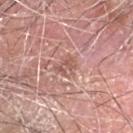{
  "patient": {
    "sex": "male",
    "age_approx": 80
  },
  "image": {
    "source": "total-body photography crop",
    "field_of_view_mm": 15
  },
  "site": "head or neck"
}